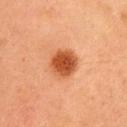Assessment:
This lesion was catalogued during total-body skin photography and was not selected for biopsy.
Clinical summary:
An algorithmic analysis of the crop reported an area of roughly 11 mm² and an outline eccentricity of about 0.4 (0 = round, 1 = elongated). And it measured a lesion color around L≈48 a*≈27 b*≈37 in CIELAB, a lesion–skin lightness drop of about 13, and a lesion-to-skin contrast of about 10 (normalized; higher = more distinct). The software also gave a border-irregularity index near 1/10, internal color variation of about 4.5 on a 0–10 scale, and radial color variation of about 1. Approximately 3.5 mm at its widest. The lesion is located on the head or neck. Imaged with cross-polarized lighting. A region of skin cropped from a whole-body photographic capture, roughly 15 mm wide. The patient is a female aged approximately 20.